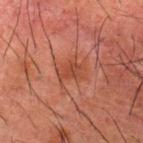biopsy_status: not biopsied; imaged during a skin examination
image:
  source: total-body photography crop
  field_of_view_mm: 15
site: upper back
lighting: cross-polarized
lesion_size:
  long_diameter_mm_approx: 3.5
patient:
  sex: male
  age_approx: 50